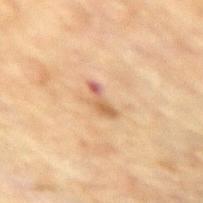<record>
  <biopsy_status>not biopsied; imaged during a skin examination</biopsy_status>
  <lighting>cross-polarized</lighting>
  <image>
    <source>total-body photography crop</source>
    <field_of_view_mm>15</field_of_view_mm>
  </image>
  <lesion_size>
    <long_diameter_mm_approx>3.0</long_diameter_mm_approx>
  </lesion_size>
  <automated_metrics>
    <area_mm2_approx>3.0</area_mm2_approx>
    <eccentricity>0.9</eccentricity>
    <shape_asymmetry>0.4</shape_asymmetry>
  </automated_metrics>
  <patient>
    <sex>female</sex>
    <age_approx>80</age_approx>
  </patient>
  <site>arm</site>
</record>